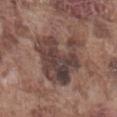biopsy status: catalogued during a skin exam; not biopsied | subject: male, aged 73–77 | body site: the abdomen | image: total-body-photography crop, ~15 mm field of view | automated lesion analysis: an average lesion color of about L≈40 a*≈16 b*≈20 (CIELAB) and about 11 CIELAB-L* units darker than the surrounding skin; an automated nevus-likeness rating near 0 out of 100 and a lesion-detection confidence of about 90/100 | size: ≈6.5 mm.